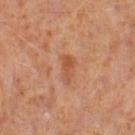Clinical summary:
Cropped from a whole-body photographic skin survey; the tile spans about 15 mm. On the left thigh. The patient is a male roughly 60 years of age.